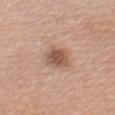Clinical impression: This lesion was catalogued during total-body skin photography and was not selected for biopsy. Acquisition and patient details: Cropped from a total-body skin-imaging series; the visible field is about 15 mm. The lesion is located on the left upper arm. A female patient roughly 55 years of age. The tile uses white-light illumination. Measured at roughly 3 mm in maximum diameter.